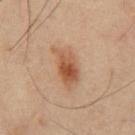Findings:
– follow-up — no biopsy performed (imaged during a skin exam)
– subject — male, aged 53–57
– body site — the chest
– image — ~15 mm crop, total-body skin-cancer survey